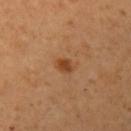Recorded during total-body skin imaging; not selected for excision or biopsy.
Located on the left upper arm.
A lesion tile, about 15 mm wide, cut from a 3D total-body photograph.
The tile uses cross-polarized illumination.
Longest diameter approximately 2 mm.
Automated tile analysis of the lesion measured a lesion area of about 3 mm² and a symmetry-axis asymmetry near 0.2. The analysis additionally found a lesion color around L≈44 a*≈24 b*≈37 in CIELAB, roughly 10 lightness units darker than nearby skin, and a lesion-to-skin contrast of about 8 (normalized; higher = more distinct). And it measured a border-irregularity rating of about 1.5/10 and peripheral color asymmetry of about 0.5. It also reported a nevus-likeness score of about 90/100 and lesion-presence confidence of about 100/100.
A female subject in their 40s.